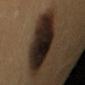biopsy_status: not biopsied; imaged during a skin examination
patient:
  sex: female
  age_approx: 30
lesion_size:
  long_diameter_mm_approx: 7.5
site: right upper arm
lighting: cross-polarized
image:
  source: total-body photography crop
  field_of_view_mm: 15